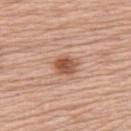  biopsy_status: not biopsied; imaged during a skin examination
  site: upper back
  lesion_size:
    long_diameter_mm_approx: 2.5
  image:
    source: total-body photography crop
    field_of_view_mm: 15
  patient:
    sex: female
    age_approx: 65